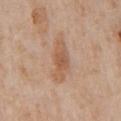Clinical impression: The lesion was photographed on a routine skin check and not biopsied; there is no pathology result. Image and clinical context: The subject is a male roughly 65 years of age. The tile uses white-light illumination. The lesion is located on the chest. The lesion's longest dimension is about 5.5 mm. A 15 mm close-up tile from a total-body photography series done for melanoma screening. An algorithmic analysis of the crop reported a lesion area of about 9.5 mm², an eccentricity of roughly 0.9, and two-axis asymmetry of about 0.3. It also reported border irregularity of about 3.5 on a 0–10 scale and a color-variation rating of about 3/10.